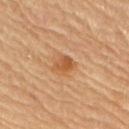Q: What is the anatomic site?
A: the arm
Q: Patient demographics?
A: male, aged 83 or older
Q: How was this image acquired?
A: 15 mm crop, total-body photography
Q: Automated lesion metrics?
A: an area of roughly 4 mm² and a shape-asymmetry score of about 0.2 (0 = symmetric); a nevus-likeness score of about 80/100 and a detector confidence of about 100 out of 100 that the crop contains a lesion
Q: What lighting was used for the tile?
A: cross-polarized